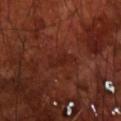| key | value |
|---|---|
| notes | no biopsy performed (imaged during a skin exam) |
| site | the front of the torso |
| image source | total-body-photography crop, ~15 mm field of view |
| lighting | cross-polarized |
| patient | male, aged 68–72 |